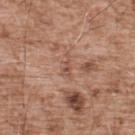Assessment: Part of a total-body skin-imaging series; this lesion was reviewed on a skin check and was not flagged for biopsy. Clinical summary: On the upper back. The recorded lesion diameter is about 3 mm. This image is a 15 mm lesion crop taken from a total-body photograph. The patient is a male aged 53–57.